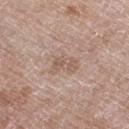• follow-up · total-body-photography surveillance lesion; no biopsy
• site · the right thigh
• subject · male, about 80 years old
• image · total-body-photography crop, ~15 mm field of view